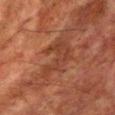Q: Was this lesion biopsied?
A: imaged on a skin check; not biopsied
Q: What did automated image analysis measure?
A: a footprint of about 11 mm², a shape eccentricity near 0.85, and a symmetry-axis asymmetry near 0.5; a border-irregularity index near 7.5/10, a color-variation rating of about 2.5/10, and peripheral color asymmetry of about 0.5; an automated nevus-likeness rating near 0 out of 100
Q: Who is the patient?
A: male, roughly 65 years of age
Q: What is the anatomic site?
A: the chest
Q: What is the imaging modality?
A: ~15 mm crop, total-body skin-cancer survey
Q: Lesion size?
A: ≈6 mm